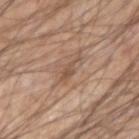Notes:
• workup · imaged on a skin check; not biopsied
• lighting · white-light illumination
• patient · male, in their mid- to late 60s
• size · ≈3.5 mm
• image · ~15 mm tile from a whole-body skin photo
• body site · the right upper arm
• TBP lesion metrics · an average lesion color of about L≈52 a*≈18 b*≈29 (CIELAB), roughly 8 lightness units darker than nearby skin, and a normalized border contrast of about 6